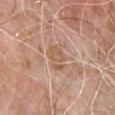This lesion was catalogued during total-body skin photography and was not selected for biopsy. The tile uses white-light illumination. Approximately 3.5 mm at its widest. The lesion is located on the front of the torso. A male patient aged around 80. Cropped from a total-body skin-imaging series; the visible field is about 15 mm.